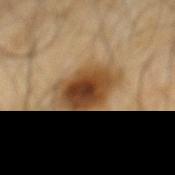Q: Was a biopsy performed?
A: imaged on a skin check; not biopsied
Q: What are the patient's age and sex?
A: male, about 65 years old
Q: How large is the lesion?
A: about 7 mm
Q: Automated lesion metrics?
A: an area of roughly 20 mm² and a symmetry-axis asymmetry near 0.2
Q: How was this image acquired?
A: ~15 mm crop, total-body skin-cancer survey
Q: Lesion location?
A: the mid back
Q: Illumination type?
A: cross-polarized illumination